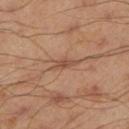The lesion was photographed on a routine skin check and not biopsied; there is no pathology result.